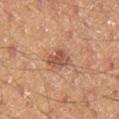Assessment: Captured during whole-body skin photography for melanoma surveillance; the lesion was not biopsied. Clinical summary: About 3.5 mm across. The subject is a male in their mid- to late 40s. Imaged with cross-polarized lighting. From the right lower leg. This image is a 15 mm lesion crop taken from a total-body photograph.